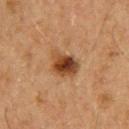Q: Is there a histopathology result?
A: no biopsy performed (imaged during a skin exam)
Q: What is the imaging modality?
A: ~15 mm crop, total-body skin-cancer survey
Q: Automated lesion metrics?
A: an area of roughly 8 mm², an outline eccentricity of about 0.6 (0 = round, 1 = elongated), and two-axis asymmetry of about 0.25; a lesion-detection confidence of about 100/100
Q: How large is the lesion?
A: ~3.5 mm (longest diameter)
Q: Who is the patient?
A: male, aged 58 to 62
Q: How was the tile lit?
A: cross-polarized illumination